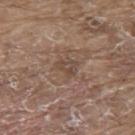Assessment:
Captured during whole-body skin photography for melanoma surveillance; the lesion was not biopsied.
Background:
From the back. The patient is a male aged approximately 80. A region of skin cropped from a whole-body photographic capture, roughly 15 mm wide.